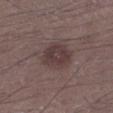Q: Was this lesion biopsied?
A: imaged on a skin check; not biopsied
Q: How was this image acquired?
A: ~15 mm crop, total-body skin-cancer survey
Q: Where on the body is the lesion?
A: the left lower leg
Q: What lighting was used for the tile?
A: white-light illumination
Q: Who is the patient?
A: male, aged around 55